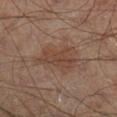Context:
A lesion tile, about 15 mm wide, cut from a 3D total-body photograph. The subject is a male aged 63–67. The lesion is on the right lower leg. Longest diameter approximately 5.5 mm.